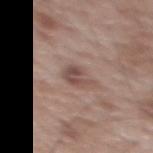Assessment:
Part of a total-body skin-imaging series; this lesion was reviewed on a skin check and was not flagged for biopsy.
Image and clinical context:
On the upper back. Captured under white-light illumination. A male patient, roughly 70 years of age. Longest diameter approximately 3.5 mm. A roughly 15 mm field-of-view crop from a total-body skin photograph. Automated image analysis of the tile measured an area of roughly 5 mm², a shape eccentricity near 0.7, and a shape-asymmetry score of about 0.3 (0 = symmetric). The software also gave a mean CIELAB color near L≈49 a*≈18 b*≈22 and a lesion-to-skin contrast of about 7.5 (normalized; higher = more distinct).